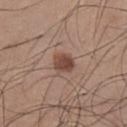Case summary:
• image: total-body-photography crop, ~15 mm field of view
• location: the chest
• patient: male, aged 23 to 27
• lighting: white-light
• diameter: ~3 mm (longest diameter)
• automated lesion analysis: a footprint of about 5.5 mm², an outline eccentricity of about 0.6 (0 = round, 1 = elongated), and two-axis asymmetry of about 0.2